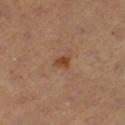Imaged during a routine full-body skin examination; the lesion was not biopsied and no histopathology is available.
About 2.5 mm across.
The subject is a female aged 38 to 42.
The tile uses cross-polarized illumination.
The lesion is on the left thigh.
The lesion-visualizer software estimated a footprint of about 3 mm² and a symmetry-axis asymmetry near 0.4. It also reported a lesion color around L≈43 a*≈21 b*≈32 in CIELAB, about 9 CIELAB-L* units darker than the surrounding skin, and a normalized border contrast of about 7.5. And it measured a nevus-likeness score of about 85/100 and a lesion-detection confidence of about 100/100.
Cropped from a whole-body photographic skin survey; the tile spans about 15 mm.